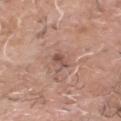Case summary:
– follow-up — imaged on a skin check; not biopsied
– body site — the head or neck
– lesion size — ≈3 mm
– acquisition — ~15 mm tile from a whole-body skin photo
– patient — male, aged 68 to 72